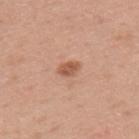imaging modality: ~15 mm crop, total-body skin-cancer survey
lighting: white-light illumination
patient: male, about 45 years old
body site: the upper back